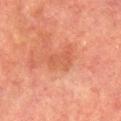The patient is a male aged 73 to 77. The lesion-visualizer software estimated an average lesion color of about L≈48 a*≈26 b*≈32 (CIELAB) and a normalized lesion–skin contrast near 4.5. And it measured border irregularity of about 4 on a 0–10 scale, a color-variation rating of about 1.5/10, and a peripheral color-asymmetry measure near 0.5. It also reported an automated nevus-likeness rating near 0 out of 100 and a lesion-detection confidence of about 100/100. The tile uses cross-polarized illumination. A region of skin cropped from a whole-body photographic capture, roughly 15 mm wide. The lesion's longest dimension is about 3.5 mm. On the right lower leg.